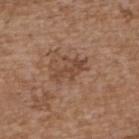• illumination — white-light
• diameter — about 4 mm
• location — the upper back
• image — ~15 mm tile from a whole-body skin photo
• subject — female, about 65 years old